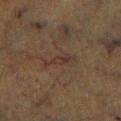biopsy_status: not biopsied; imaged during a skin examination
image:
  source: total-body photography crop
  field_of_view_mm: 15
patient:
  sex: male
  age_approx: 75
site: right lower leg
lighting: cross-polarized
automated_metrics:
  cielab_L: 26
  cielab_a: 13
  cielab_b: 18
  vs_skin_darker_L: 4.0
  vs_skin_contrast_norm: 6.0
  border_irregularity_0_10: 4.0
  color_variation_0_10: 2.5
  peripheral_color_asymmetry: 0.5